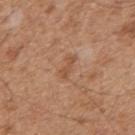{"biopsy_status": "not biopsied; imaged during a skin examination", "lesion_size": {"long_diameter_mm_approx": 3.0}, "image": {"source": "total-body photography crop", "field_of_view_mm": 15}, "lighting": "white-light", "automated_metrics": {"area_mm2_approx": 2.5, "eccentricity": 0.95, "shape_asymmetry": 0.4, "vs_skin_darker_L": 8.0, "vs_skin_contrast_norm": 6.0, "border_irregularity_0_10": 5.0, "color_variation_0_10": 0.0}, "site": "upper back", "patient": {"sex": "male", "age_approx": 45}}